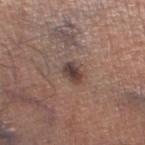Part of a total-body skin-imaging series; this lesion was reviewed on a skin check and was not flagged for biopsy.
The lesion is located on the left thigh.
A roughly 15 mm field-of-view crop from a total-body skin photograph.
The total-body-photography lesion software estimated a footprint of about 4 mm² and a shape eccentricity near 0.7. The software also gave a classifier nevus-likeness of about 80/100 and a detector confidence of about 100 out of 100 that the crop contains a lesion.
Measured at roughly 2.5 mm in maximum diameter.
The patient is a male about 70 years old.
This is a white-light tile.